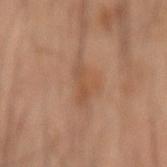acquisition: ~15 mm crop, total-body skin-cancer survey | lesion size: ≈5 mm | lighting: cross-polarized illumination | patient: male, roughly 65 years of age | body site: the right forearm.